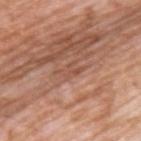• notes · no biopsy performed (imaged during a skin exam)
• illumination · white-light
• site · the upper back
• subject · male, approximately 60 years of age
• acquisition · total-body-photography crop, ~15 mm field of view